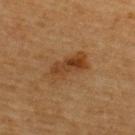No biopsy was performed on this lesion — it was imaged during a full skin examination and was not determined to be concerning.
Located on the back.
A close-up tile cropped from a whole-body skin photograph, about 15 mm across.
The patient is a male in their mid- to late 60s.